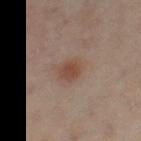Impression: Captured during whole-body skin photography for melanoma surveillance; the lesion was not biopsied. Acquisition and patient details: About 3 mm across. The patient is a female aged 38–42. The lesion is located on the right leg. Captured under cross-polarized illumination. This image is a 15 mm lesion crop taken from a total-body photograph.